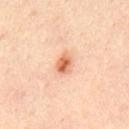Clinical impression:
Captured during whole-body skin photography for melanoma surveillance; the lesion was not biopsied.
Background:
The lesion is located on the upper back. Captured under cross-polarized illumination. Measured at roughly 2.5 mm in maximum diameter. A male subject, aged 33 to 37. This image is a 15 mm lesion crop taken from a total-body photograph.